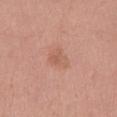<lesion>
  <biopsy_status>not biopsied; imaged during a skin examination</biopsy_status>
  <lighting>white-light</lighting>
  <lesion_size>
    <long_diameter_mm_approx>3.0</long_diameter_mm_approx>
  </lesion_size>
  <image>
    <source>total-body photography crop</source>
    <field_of_view_mm>15</field_of_view_mm>
  </image>
  <patient>
    <sex>female</sex>
    <age_approx>65</age_approx>
  </patient>
  <automated_metrics>
    <border_irregularity_0_10>3.5</border_irregularity_0_10>
    <color_variation_0_10>2.0</color_variation_0_10>
    <peripheral_color_asymmetry>0.5</peripheral_color_asymmetry>
    <nevus_likeness_0_100>5</nevus_likeness_0_100>
  </automated_metrics>
  <site>left thigh</site>
</lesion>